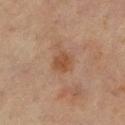{
  "biopsy_status": "not biopsied; imaged during a skin examination",
  "site": "left lower leg",
  "lesion_size": {
    "long_diameter_mm_approx": 2.5
  },
  "image": {
    "source": "total-body photography crop",
    "field_of_view_mm": 15
  },
  "patient": {
    "sex": "female",
    "age_approx": 55
  }
}